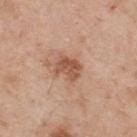follow-up: total-body-photography surveillance lesion; no biopsy
illumination: white-light
image-analysis metrics: a footprint of about 7 mm², an eccentricity of roughly 0.6, and a shape-asymmetry score of about 0.2 (0 = symmetric); an average lesion color of about L≈54 a*≈23 b*≈32 (CIELAB) and a lesion–skin lightness drop of about 11; a nevus-likeness score of about 70/100 and lesion-presence confidence of about 100/100
site: the upper back
subject: male, in their mid-50s
acquisition: 15 mm crop, total-body photography